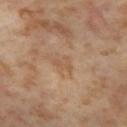Part of a total-body skin-imaging series; this lesion was reviewed on a skin check and was not flagged for biopsy.
An algorithmic analysis of the crop reported an area of roughly 2.5 mm², an eccentricity of roughly 0.8, and a symmetry-axis asymmetry near 0.55. The analysis additionally found about 6 CIELAB-L* units darker than the surrounding skin and a normalized border contrast of about 4.5. It also reported a classifier nevus-likeness of about 0/100.
A roughly 15 mm field-of-view crop from a total-body skin photograph.
Longest diameter approximately 2.5 mm.
From the left thigh.
Imaged with cross-polarized lighting.
A female patient about 55 years old.